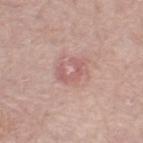No biopsy was performed on this lesion — it was imaged during a full skin examination and was not determined to be concerning. The total-body-photography lesion software estimated a lesion area of about 4 mm² and a shape eccentricity near 0.8. And it measured a border-irregularity rating of about 8/10, a within-lesion color-variation index near 0/10, and radial color variation of about 0. The lesion's longest dimension is about 3 mm. The lesion is located on the leg. A female patient, aged 63 to 67. The tile uses white-light illumination. A close-up tile cropped from a whole-body skin photograph, about 15 mm across.